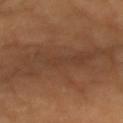Captured during whole-body skin photography for melanoma surveillance; the lesion was not biopsied.
A 15 mm close-up tile from a total-body photography series done for melanoma screening.
The lesion is located on the arm.
The total-body-photography lesion software estimated a footprint of about 85 mm², an eccentricity of roughly 0.85, and a shape-asymmetry score of about 0.35 (0 = symmetric). The software also gave an average lesion color of about L≈43 a*≈19 b*≈30 (CIELAB) and about 7 CIELAB-L* units darker than the surrounding skin. And it measured a border-irregularity index near 7.5/10, a within-lesion color-variation index near 4/10, and peripheral color asymmetry of about 1.
Longest diameter approximately 15 mm.
Captured under cross-polarized illumination.
A female patient roughly 55 years of age.